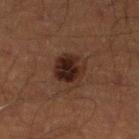The lesion was photographed on a routine skin check and not biopsied; there is no pathology result.
The subject is a male aged around 45.
Cropped from a whole-body photographic skin survey; the tile spans about 15 mm.
The lesion is located on the left lower leg.